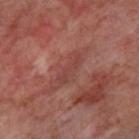{"biopsy_status": "not biopsied; imaged during a skin examination", "patient": {"age_approx": 55}, "image": {"source": "total-body photography crop", "field_of_view_mm": 15}, "lesion_size": {"long_diameter_mm_approx": 4.0}, "site": "upper back", "lighting": "cross-polarized"}